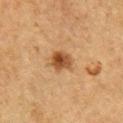<tbp_lesion>
  <biopsy_status>not biopsied; imaged during a skin examination</biopsy_status>
  <patient>
    <sex>male</sex>
    <age_approx>75</age_approx>
  </patient>
  <lesion_size>
    <long_diameter_mm_approx>3.5</long_diameter_mm_approx>
  </lesion_size>
  <automated_metrics>
    <area_mm2_approx>6.0</area_mm2_approx>
    <eccentricity>0.65</eccentricity>
    <shape_asymmetry>0.2</shape_asymmetry>
    <border_irregularity_0_10>2.5</border_irregularity_0_10>
    <color_variation_0_10>5.5</color_variation_0_10>
    <peripheral_color_asymmetry>1.5</peripheral_color_asymmetry>
    <lesion_detection_confidence_0_100>100</lesion_detection_confidence_0_100>
  </automated_metrics>
  <lighting>cross-polarized</lighting>
  <image>
    <source>total-body photography crop</source>
    <field_of_view_mm>15</field_of_view_mm>
  </image>
  <site>front of the torso</site>
</tbp_lesion>